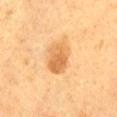biopsy status = total-body-photography surveillance lesion; no biopsy | imaging modality = ~15 mm tile from a whole-body skin photo | lesion diameter = about 4 mm | site = the abdomen | automated lesion analysis = a footprint of about 9.5 mm², an eccentricity of roughly 0.75, and a symmetry-axis asymmetry near 0.15; a border-irregularity rating of about 1.5/10, a within-lesion color-variation index near 4/10, and a peripheral color-asymmetry measure near 1.5 | subject = male, in their mid- to late 80s or older.